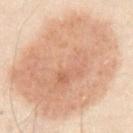| key | value |
|---|---|
| workup | imaged on a skin check; not biopsied |
| subject | male, in their 50s |
| tile lighting | cross-polarized |
| TBP lesion metrics | a footprint of about 110 mm² and an outline eccentricity of about 0.45 (0 = round, 1 = elongated); an average lesion color of about L≈56 a*≈15 b*≈27 (CIELAB), roughly 10 lightness units darker than nearby skin, and a normalized lesion–skin contrast near 7 |
| size | ~12.5 mm (longest diameter) |
| image source | 15 mm crop, total-body photography |
| site | the abdomen |